Located on the chest · a male subject approximately 50 years of age · a lesion tile, about 15 mm wide, cut from a 3D total-body photograph: 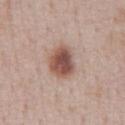Q: What lighting was used for the tile?
A: white-light illumination
Q: What did pathology find?
A: a dysplastic (Clark) nevus